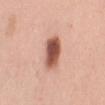Clinical impression:
Part of a total-body skin-imaging series; this lesion was reviewed on a skin check and was not flagged for biopsy.
Background:
A lesion tile, about 15 mm wide, cut from a 3D total-body photograph. From the arm. Automated tile analysis of the lesion measured an average lesion color of about L≈54 a*≈25 b*≈29 (CIELAB), about 18 CIELAB-L* units darker than the surrounding skin, and a normalized lesion–skin contrast near 11.5. It also reported a nevus-likeness score of about 95/100 and lesion-presence confidence of about 100/100. The subject is a female about 50 years old. The tile uses white-light illumination. Measured at roughly 3.5 mm in maximum diameter.